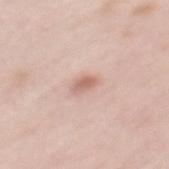Clinical summary: This is a white-light tile. A female subject, aged 63 to 67. The lesion-visualizer software estimated an area of roughly 3 mm² and a shape eccentricity near 0.85. It also reported a lesion color around L≈64 a*≈20 b*≈26 in CIELAB, a lesion–skin lightness drop of about 11, and a normalized border contrast of about 6.5. The analysis additionally found border irregularity of about 3 on a 0–10 scale and peripheral color asymmetry of about 1. The analysis additionally found an automated nevus-likeness rating near 75 out of 100. The recorded lesion diameter is about 2.5 mm. On the mid back. A region of skin cropped from a whole-body photographic capture, roughly 15 mm wide.